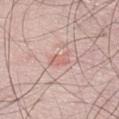{"biopsy_status": "not biopsied; imaged during a skin examination", "site": "abdomen", "lesion_size": {"long_diameter_mm_approx": 2.5}, "image": {"source": "total-body photography crop", "field_of_view_mm": 15}, "patient": {"sex": "male", "age_approx": "85+"}, "automated_metrics": {"eccentricity": 0.85, "shape_asymmetry": 0.3, "lesion_detection_confidence_0_100": 100}, "lighting": "white-light"}